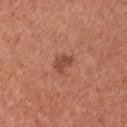biopsy_status: not biopsied; imaged during a skin examination
automated_metrics:
  nevus_likeness_0_100: 45
  lesion_detection_confidence_0_100: 100
site: front of the torso
patient:
  sex: female
  age_approx: 35
lighting: white-light
image:
  source: total-body photography crop
  field_of_view_mm: 15
lesion_size:
  long_diameter_mm_approx: 2.5